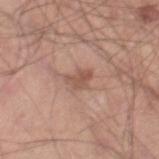Q: Was this lesion biopsied?
A: imaged on a skin check; not biopsied
Q: Lesion location?
A: the right thigh
Q: Who is the patient?
A: male, about 45 years old
Q: How was this image acquired?
A: 15 mm crop, total-body photography